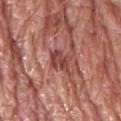Captured during whole-body skin photography for melanoma surveillance; the lesion was not biopsied.
Imaged with white-light lighting.
The recorded lesion diameter is about 3 mm.
Located on the left lower leg.
A male subject in their 80s.
Cropped from a whole-body photographic skin survey; the tile spans about 15 mm.